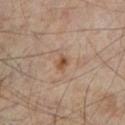follow-up=catalogued during a skin exam; not biopsied | subject=male, aged around 65 | lighting=cross-polarized illumination | site=the left lower leg | automated metrics=a lesion color around L≈48 a*≈18 b*≈31 in CIELAB, roughly 9 lightness units darker than nearby skin, and a normalized border contrast of about 8; a border-irregularity index near 1.5/10, a color-variation rating of about 4/10, and radial color variation of about 1.5 | lesion size=~2 mm (longest diameter) | imaging modality=~15 mm crop, total-body skin-cancer survey.